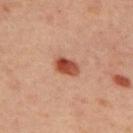body site: the upper back | subject: female, roughly 45 years of age | image: ~15 mm crop, total-body skin-cancer survey | size: ≈3 mm.